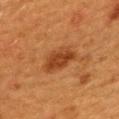| key | value |
|---|---|
| diameter | ~4.5 mm (longest diameter) |
| lighting | cross-polarized illumination |
| subject | female, aged around 40 |
| body site | the mid back |
| image source | ~15 mm tile from a whole-body skin photo |